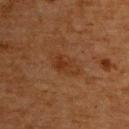Q: Is there a histopathology result?
A: catalogued during a skin exam; not biopsied